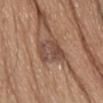follow-up: no biopsy performed (imaged during a skin exam) | TBP lesion metrics: an automated nevus-likeness rating near 0 out of 100 and a detector confidence of about 50 out of 100 that the crop contains a lesion | imaging modality: ~15 mm tile from a whole-body skin photo | location: the chest | subject: female, in their mid-60s | lesion size: ~4.5 mm (longest diameter) | illumination: white-light illumination.